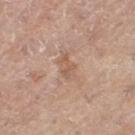The lesion was photographed on a routine skin check and not biopsied; there is no pathology result. A close-up tile cropped from a whole-body skin photograph, about 15 mm across. A female subject aged approximately 65. The lesion is located on the left thigh.